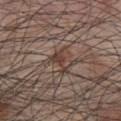Impression:
This lesion was catalogued during total-body skin photography and was not selected for biopsy.
Background:
The lesion is on the chest. A roughly 15 mm field-of-view crop from a total-body skin photograph. A male patient, aged 48 to 52. This is a white-light tile.